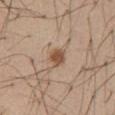{
  "biopsy_status": "not biopsied; imaged during a skin examination",
  "automated_metrics": {
    "nevus_likeness_0_100": 95,
    "lesion_detection_confidence_0_100": 100
  },
  "patient": {
    "sex": "male",
    "age_approx": 55
  },
  "site": "abdomen",
  "lighting": "white-light",
  "image": {
    "source": "total-body photography crop",
    "field_of_view_mm": 15
  },
  "lesion_size": {
    "long_diameter_mm_approx": 3.0
  }
}